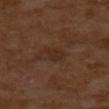workup = catalogued during a skin exam; not biopsied | subject = female, about 55 years old | image = ~15 mm crop, total-body skin-cancer survey | location = the upper back.